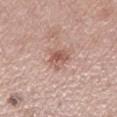notes: imaged on a skin check; not biopsied | subject: male, aged 63–67 | tile lighting: white-light | image-analysis metrics: an average lesion color of about L≈56 a*≈22 b*≈25 (CIELAB), about 11 CIELAB-L* units darker than the surrounding skin, and a normalized border contrast of about 7 | acquisition: ~15 mm tile from a whole-body skin photo | size: ≈3 mm | location: the left lower leg.